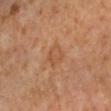{
  "site": "left forearm",
  "lighting": "cross-polarized",
  "lesion_size": {
    "long_diameter_mm_approx": 2.5
  },
  "image": {
    "source": "total-body photography crop",
    "field_of_view_mm": 15
  },
  "patient": {
    "sex": "female",
    "age_approx": 50
  }
}